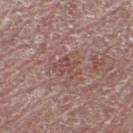Clinical summary: Imaged with white-light lighting. Automated image analysis of the tile measured a footprint of about 5.5 mm². It also reported a mean CIELAB color near L≈48 a*≈20 b*≈22, a lesion–skin lightness drop of about 7, and a normalized lesion–skin contrast near 5.5. And it measured border irregularity of about 7.5 on a 0–10 scale, a color-variation rating of about 2.5/10, and radial color variation of about 1. And it measured a nevus-likeness score of about 0/100 and a detector confidence of about 75 out of 100 that the crop contains a lesion. Measured at roughly 3 mm in maximum diameter. The lesion is located on the left thigh. This image is a 15 mm lesion crop taken from a total-body photograph. A female patient approximately 65 years of age.